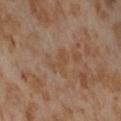anatomic site — the front of the torso | TBP lesion metrics — a lesion area of about 3.5 mm², a shape eccentricity near 0.85, and a symmetry-axis asymmetry near 0.35; a lesion color around L≈48 a*≈17 b*≈31 in CIELAB, roughly 5 lightness units darker than nearby skin, and a normalized border contrast of about 5 | imaging modality — ~15 mm crop, total-body skin-cancer survey | lighting — cross-polarized illumination | patient — female, aged 53 to 57.